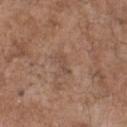Assessment: This lesion was catalogued during total-body skin photography and was not selected for biopsy. Clinical summary: Measured at roughly 2.5 mm in maximum diameter. A male patient, aged 68 to 72. An algorithmic analysis of the crop reported a border-irregularity index near 5/10, a color-variation rating of about 0/10, and radial color variation of about 0. From the chest. A 15 mm close-up extracted from a 3D total-body photography capture. This is a white-light tile.